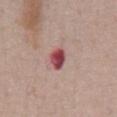The total-body-photography lesion software estimated border irregularity of about 2 on a 0–10 scale, a within-lesion color-variation index near 5/10, and a peripheral color-asymmetry measure near 1.5.
Captured under white-light illumination.
Measured at roughly 2.5 mm in maximum diameter.
A male patient in their mid-50s.
Cropped from a total-body skin-imaging series; the visible field is about 15 mm.
From the abdomen.